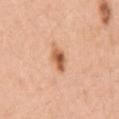The lesion was photographed on a routine skin check and not biopsied; there is no pathology result. Automated tile analysis of the lesion measured an area of roughly 5 mm², an outline eccentricity of about 0.75 (0 = round, 1 = elongated), and a symmetry-axis asymmetry near 0.15. And it measured a lesion color around L≈60 a*≈25 b*≈37 in CIELAB and a normalized lesion–skin contrast near 9. The software also gave a classifier nevus-likeness of about 95/100 and a detector confidence of about 100 out of 100 that the crop contains a lesion. A female subject about 45 years old. A 15 mm close-up extracted from a 3D total-body photography capture. Located on the left upper arm.